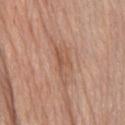A 15 mm crop from a total-body photograph taken for skin-cancer surveillance. The lesion is on the mid back. About 4.5 mm across. This is a white-light tile. The subject is a male in their 80s.